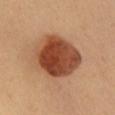This lesion was catalogued during total-body skin photography and was not selected for biopsy. A 15 mm close-up extracted from a 3D total-body photography capture. On the chest. The recorded lesion diameter is about 6 mm. The subject is a female aged around 35. Captured under cross-polarized illumination.